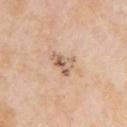This lesion was catalogued during total-body skin photography and was not selected for biopsy.
A female subject in their 60s.
Located on the arm.
Approximately 3 mm at its widest.
Captured under white-light illumination.
Cropped from a whole-body photographic skin survey; the tile spans about 15 mm.